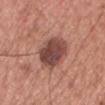Assessment:
No biopsy was performed on this lesion — it was imaged during a full skin examination and was not determined to be concerning.
Image and clinical context:
The tile uses white-light illumination. The subject is a male aged around 40. The total-body-photography lesion software estimated a lesion area of about 16 mm², an eccentricity of roughly 0.75, and two-axis asymmetry of about 0.15. The software also gave an average lesion color of about L≈46 a*≈23 b*≈25 (CIELAB) and a lesion–skin lightness drop of about 14. The software also gave a nevus-likeness score of about 60/100. A 15 mm close-up tile from a total-body photography series done for melanoma screening. On the head or neck.